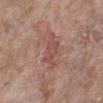biopsy status: total-body-photography surveillance lesion; no biopsy | body site: the left lower leg | lesion size: ≈4.5 mm | subject: female, aged 73 to 77 | acquisition: total-body-photography crop, ~15 mm field of view | illumination: white-light illumination.